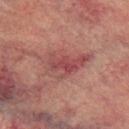Part of a total-body skin-imaging series; this lesion was reviewed on a skin check and was not flagged for biopsy. The lesion is located on the right lower leg. Automated tile analysis of the lesion measured a footprint of about 13 mm², an outline eccentricity of about 0.8 (0 = round, 1 = elongated), and two-axis asymmetry of about 0.35. The software also gave a nevus-likeness score of about 0/100 and a lesion-detection confidence of about 75/100. A close-up tile cropped from a whole-body skin photograph, about 15 mm across. The lesion's longest dimension is about 6 mm. A male patient, aged 73–77. Captured under cross-polarized illumination.